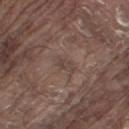Imaged during a routine full-body skin examination; the lesion was not biopsied and no histopathology is available. A 15 mm close-up tile from a total-body photography series done for melanoma screening. On the right thigh. A male subject, aged around 80.